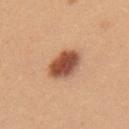Background:
Located on the back. A region of skin cropped from a whole-body photographic capture, roughly 15 mm wide. The subject is a female about 25 years old. The recorded lesion diameter is about 4.5 mm.